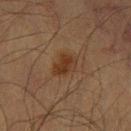follow-up=total-body-photography surveillance lesion; no biopsy | illumination=cross-polarized illumination | imaging modality=total-body-photography crop, ~15 mm field of view | site=the right thigh | patient=male, about 65 years old.